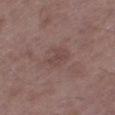<lesion>
  <biopsy_status>not biopsied; imaged during a skin examination</biopsy_status>
  <lesion_size>
    <long_diameter_mm_approx>3.5</long_diameter_mm_approx>
  </lesion_size>
  <lighting>white-light</lighting>
  <image>
    <source>total-body photography crop</source>
    <field_of_view_mm>15</field_of_view_mm>
  </image>
  <patient>
    <sex>male</sex>
    <age_approx>50</age_approx>
  </patient>
  <site>right thigh</site>
</lesion>